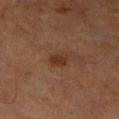A close-up tile cropped from a whole-body skin photograph, about 15 mm across. On the left lower leg. The patient is a male about 65 years old.